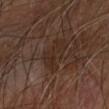About 4.5 mm across. A male patient, aged approximately 70. Imaged with cross-polarized lighting. Located on the left forearm. Automated tile analysis of the lesion measured a footprint of about 8.5 mm², an outline eccentricity of about 0.8 (0 = round, 1 = elongated), and a symmetry-axis asymmetry near 0.45. And it measured a border-irregularity index near 6/10. A region of skin cropped from a whole-body photographic capture, roughly 15 mm wide.